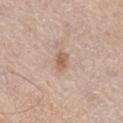notes: no biopsy performed (imaged during a skin exam); site: the right thigh; patient: male, in their mid-70s; acquisition: total-body-photography crop, ~15 mm field of view.